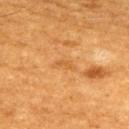| field | value |
|---|---|
| workup | catalogued during a skin exam; not biopsied |
| subject | male, aged approximately 60 |
| body site | the upper back |
| illumination | cross-polarized illumination |
| imaging modality | ~15 mm tile from a whole-body skin photo |
| TBP lesion metrics | a color-variation rating of about 0/10 and radial color variation of about 0; an automated nevus-likeness rating near 0 out of 100 |